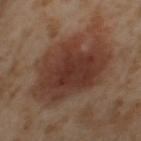Q: Was a biopsy performed?
A: imaged on a skin check; not biopsied
Q: What is the imaging modality?
A: ~15 mm tile from a whole-body skin photo
Q: Automated lesion metrics?
A: an eccentricity of roughly 0.8; an average lesion color of about L≈36 a*≈20 b*≈25 (CIELAB), a lesion–skin lightness drop of about 10, and a normalized lesion–skin contrast near 9; a border-irregularity index near 4/10 and radial color variation of about 1; a classifier nevus-likeness of about 80/100 and lesion-presence confidence of about 100/100
Q: What is the lesion's diameter?
A: ~10 mm (longest diameter)
Q: Illumination type?
A: cross-polarized illumination
Q: Lesion location?
A: the left thigh
Q: What are the patient's age and sex?
A: female, aged 53–57